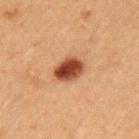Recorded during total-body skin imaging; not selected for excision or biopsy. A male subject, roughly 50 years of age. This image is a 15 mm lesion crop taken from a total-body photograph. Automated image analysis of the tile measured a border-irregularity rating of about 1.5/10, a within-lesion color-variation index near 5.5/10, and peripheral color asymmetry of about 1.5. The analysis additionally found a classifier nevus-likeness of about 100/100. This is a cross-polarized tile. The lesion is on the left upper arm. Longest diameter approximately 3.5 mm.